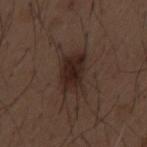This lesion was catalogued during total-body skin photography and was not selected for biopsy.
Cropped from a whole-body photographic skin survey; the tile spans about 15 mm.
Captured under white-light illumination.
The lesion is located on the mid back.
A male subject roughly 50 years of age.
About 4.5 mm across.